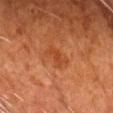Part of a total-body skin-imaging series; this lesion was reviewed on a skin check and was not flagged for biopsy. A 15 mm close-up tile from a total-body photography series done for melanoma screening. A male subject about 80 years old. Imaged with cross-polarized lighting. The lesion's longest dimension is about 3 mm. Located on the chest.